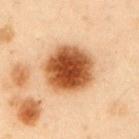Q: Was a biopsy performed?
A: total-body-photography surveillance lesion; no biopsy
Q: What is the anatomic site?
A: the left upper arm
Q: Automated lesion metrics?
A: radial color variation of about 1; a detector confidence of about 100 out of 100 that the crop contains a lesion
Q: Illumination type?
A: cross-polarized illumination
Q: What is the imaging modality?
A: ~15 mm crop, total-body skin-cancer survey
Q: What are the patient's age and sex?
A: male, aged around 55
Q: Lesion size?
A: ~6 mm (longest diameter)